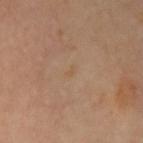Q: Lesion location?
A: the left upper arm
Q: Automated lesion metrics?
A: an eccentricity of roughly 0.8 and two-axis asymmetry of about 0.35; an average lesion color of about L≈55 a*≈16 b*≈33 (CIELAB), a lesion–skin lightness drop of about 3, and a normalized lesion–skin contrast near 4
Q: Illumination type?
A: cross-polarized illumination
Q: How was this image acquired?
A: ~15 mm crop, total-body skin-cancer survey
Q: Patient demographics?
A: female, aged 63 to 67
Q: Lesion size?
A: about 2 mm